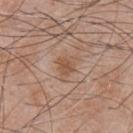Clinical impression: The lesion was tiled from a total-body skin photograph and was not biopsied. Image and clinical context: A male patient about 50 years old. The tile uses white-light illumination. Measured at roughly 3 mm in maximum diameter. A region of skin cropped from a whole-body photographic capture, roughly 15 mm wide. The lesion is on the chest.